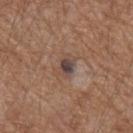Captured during whole-body skin photography for melanoma surveillance; the lesion was not biopsied. A roughly 15 mm field-of-view crop from a total-body skin photograph. The lesion is located on the left forearm. The patient is a male roughly 55 years of age. The recorded lesion diameter is about 3 mm. This is a white-light tile.